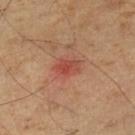No biopsy was performed on this lesion — it was imaged during a full skin examination and was not determined to be concerning. A 15 mm close-up extracted from a 3D total-body photography capture. The recorded lesion diameter is about 3 mm. From the left lower leg. This is a cross-polarized tile. A male subject aged around 55.